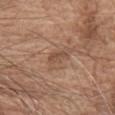Impression: The lesion was photographed on a routine skin check and not biopsied; there is no pathology result. Clinical summary: A 15 mm crop from a total-body photograph taken for skin-cancer surveillance. Imaged with white-light lighting. On the chest. A male subject, aged 73 to 77.